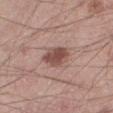<record>
  <automated_metrics>
    <cielab_L>49</cielab_L>
    <cielab_a>20</cielab_a>
    <cielab_b>23</cielab_b>
    <vs_skin_darker_L>12.0</vs_skin_darker_L>
    <vs_skin_contrast_norm>8.5</vs_skin_contrast_norm>
    <border_irregularity_0_10>2.0</border_irregularity_0_10>
    <color_variation_0_10>3.0</color_variation_0_10>
    <peripheral_color_asymmetry>1.0</peripheral_color_asymmetry>
  </automated_metrics>
  <image>
    <source>total-body photography crop</source>
    <field_of_view_mm>15</field_of_view_mm>
  </image>
  <site>left lower leg</site>
  <lighting>white-light</lighting>
  <lesion_size>
    <long_diameter_mm_approx>4.0</long_diameter_mm_approx>
  </lesion_size>
  <patient>
    <sex>male</sex>
    <age_approx>40</age_approx>
  </patient>
</record>